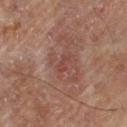Q: Was this lesion biopsied?
A: total-body-photography surveillance lesion; no biopsy
Q: Patient demographics?
A: male, aged 63–67
Q: What did automated image analysis measure?
A: a nevus-likeness score of about 0/100 and a detector confidence of about 100 out of 100 that the crop contains a lesion
Q: How large is the lesion?
A: ≈2.5 mm
Q: How was this image acquired?
A: ~15 mm crop, total-body skin-cancer survey
Q: Illumination type?
A: cross-polarized
Q: Lesion location?
A: the left thigh